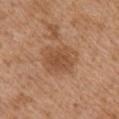Clinical impression:
Recorded during total-body skin imaging; not selected for excision or biopsy.
Acquisition and patient details:
On the right upper arm. A male patient in their mid-70s. Longest diameter approximately 4.5 mm. Automated image analysis of the tile measured a mean CIELAB color near L≈51 a*≈21 b*≈34, roughly 9 lightness units darker than nearby skin, and a normalized lesion–skin contrast near 6.5. And it measured a border-irregularity rating of about 2/10, a color-variation rating of about 2.5/10, and peripheral color asymmetry of about 1. The analysis additionally found a nevus-likeness score of about 25/100. A roughly 15 mm field-of-view crop from a total-body skin photograph.